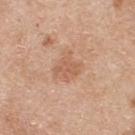<record>
<biopsy_status>not biopsied; imaged during a skin examination</biopsy_status>
<image>
  <source>total-body photography crop</source>
  <field_of_view_mm>15</field_of_view_mm>
</image>
<site>upper back</site>
<lesion_size>
  <long_diameter_mm_approx>3.5</long_diameter_mm_approx>
</lesion_size>
<lighting>white-light</lighting>
<patient>
  <sex>male</sex>
  <age_approx>60</age_approx>
</patient>
</record>